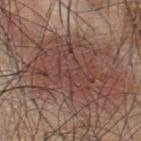No biopsy was performed on this lesion — it was imaged during a full skin examination and was not determined to be concerning.
A roughly 15 mm field-of-view crop from a total-body skin photograph.
Measured at roughly 11.5 mm in maximum diameter.
Located on the upper back.
The subject is a male aged 43–47.
Captured under white-light illumination.